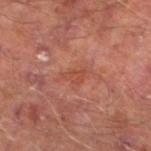  biopsy_status: not biopsied; imaged during a skin examination
  image:
    source: total-body photography crop
    field_of_view_mm: 15
  site: left lower leg
  lesion_size:
    long_diameter_mm_approx: 2.5
  patient:
    sex: male
    age_approx: 65
  lighting: cross-polarized